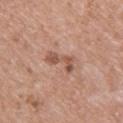<record>
<biopsy_status>not biopsied; imaged during a skin examination</biopsy_status>
<lesion_size>
  <long_diameter_mm_approx>4.5</long_diameter_mm_approx>
</lesion_size>
<patient>
  <sex>female</sex>
  <age_approx>40</age_approx>
</patient>
<lighting>white-light</lighting>
<site>upper back</site>
<image>
  <source>total-body photography crop</source>
  <field_of_view_mm>15</field_of_view_mm>
</image>
</record>